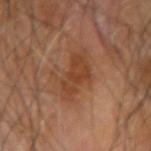Q: Was this lesion biopsied?
A: catalogued during a skin exam; not biopsied
Q: Who is the patient?
A: male, aged around 65
Q: What is the imaging modality?
A: ~15 mm tile from a whole-body skin photo
Q: Automated lesion metrics?
A: roughly 8 lightness units darker than nearby skin and a normalized lesion–skin contrast near 7; border irregularity of about 5 on a 0–10 scale, internal color variation of about 3 on a 0–10 scale, and radial color variation of about 1; a classifier nevus-likeness of about 10/100 and a detector confidence of about 100 out of 100 that the crop contains a lesion
Q: Illumination type?
A: cross-polarized illumination
Q: Lesion location?
A: the arm